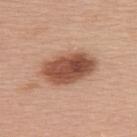workup = catalogued during a skin exam; not biopsied
illumination = white-light
automated metrics = an area of roughly 20 mm², an eccentricity of roughly 0.8, and a symmetry-axis asymmetry near 0.15; a border-irregularity index near 1.5/10 and peripheral color asymmetry of about 2; an automated nevus-likeness rating near 95 out of 100
image source = total-body-photography crop, ~15 mm field of view
patient = female, aged 58–62
location = the upper back